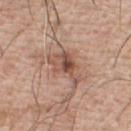Captured during whole-body skin photography for melanoma surveillance; the lesion was not biopsied. A lesion tile, about 15 mm wide, cut from a 3D total-body photograph. A male subject aged around 75. Located on the front of the torso.